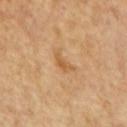Imaged during a routine full-body skin examination; the lesion was not biopsied and no histopathology is available.
This is a cross-polarized tile.
A 15 mm crop from a total-body photograph taken for skin-cancer surveillance.
The recorded lesion diameter is about 3 mm.
A male patient aged approximately 65.